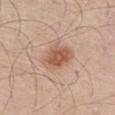{"biopsy_status": "not biopsied; imaged during a skin examination", "patient": {"sex": "male", "age_approx": 60}, "image": {"source": "total-body photography crop", "field_of_view_mm": 15}, "lighting": "white-light", "lesion_size": {"long_diameter_mm_approx": 4.5}, "site": "leg"}